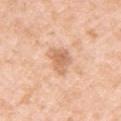Impression: No biopsy was performed on this lesion — it was imaged during a full skin examination and was not determined to be concerning. Image and clinical context: The subject is a female in their 40s. Cropped from a whole-body photographic skin survey; the tile spans about 15 mm. On the left upper arm. The tile uses white-light illumination. Measured at roughly 3.5 mm in maximum diameter.